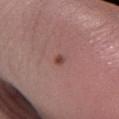Cropped from a whole-body photographic skin survey; the tile spans about 15 mm. The lesion is on the left lower leg. The patient is a female aged 23–27. Longest diameter approximately 1.5 mm.